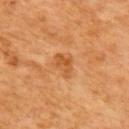biopsy status: no biopsy performed (imaged during a skin exam)
image-analysis metrics: two-axis asymmetry of about 0.35; an average lesion color of about L≈54 a*≈27 b*≈44 (CIELAB) and roughly 9 lightness units darker than nearby skin
image source: ~15 mm tile from a whole-body skin photo
size: about 2.5 mm
lighting: cross-polarized illumination
patient: female, roughly 65 years of age
anatomic site: the upper back